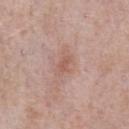Clinical impression: Recorded during total-body skin imaging; not selected for excision or biopsy. Acquisition and patient details: A roughly 15 mm field-of-view crop from a total-body skin photograph. A male patient, about 55 years old. The lesion is located on the chest.